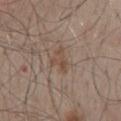The lesion was tiled from a total-body skin photograph and was not biopsied.
The total-body-photography lesion software estimated an area of roughly 4.5 mm² and a symmetry-axis asymmetry near 0.55. The analysis additionally found a lesion color around L≈48 a*≈16 b*≈26 in CIELAB and a lesion–skin lightness drop of about 7. The analysis additionally found border irregularity of about 6 on a 0–10 scale.
The patient is a male aged approximately 55.
The lesion is on the mid back.
Cropped from a whole-body photographic skin survey; the tile spans about 15 mm.